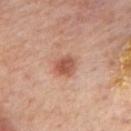Impression: The lesion was photographed on a routine skin check and not biopsied; there is no pathology result. Acquisition and patient details: Approximately 3 mm at its widest. Captured under cross-polarized illumination. A male patient, about 65 years old. On the mid back. A close-up tile cropped from a whole-body skin photograph, about 15 mm across.